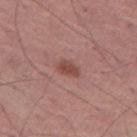biopsy_status: not biopsied; imaged during a skin examination
lighting: white-light
patient:
  sex: male
  age_approx: 70
image:
  source: total-body photography crop
  field_of_view_mm: 15
site: leg